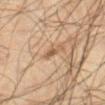The lesion was tiled from a total-body skin photograph and was not biopsied. From the right thigh. A close-up tile cropped from a whole-body skin photograph, about 15 mm across. The tile uses cross-polarized illumination. The subject is a male about 55 years old.